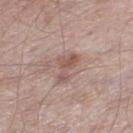Findings:
• follow-up — no biopsy performed (imaged during a skin exam)
• subject — male, aged 53–57
• body site — the leg
• lesion diameter — ≈4 mm
• TBP lesion metrics — a shape eccentricity near 0.85 and two-axis asymmetry of about 0.5; a lesion color around L≈54 a*≈19 b*≈23 in CIELAB, about 9 CIELAB-L* units darker than the surrounding skin, and a normalized lesion–skin contrast near 6.5
• acquisition — 15 mm crop, total-body photography